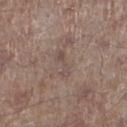patient: male, aged 63–67 | body site: the leg | image source: total-body-photography crop, ~15 mm field of view.